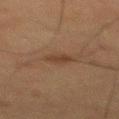follow-up = no biopsy performed (imaged during a skin exam); tile lighting = cross-polarized illumination; lesion size = about 2.5 mm; patient = male, aged approximately 60; image source = ~15 mm tile from a whole-body skin photo; site = the back.